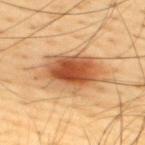Notes:
* biopsy status: imaged on a skin check; not biopsied
* subject: male, aged 43 to 47
* acquisition: ~15 mm tile from a whole-body skin photo
* automated lesion analysis: a lesion area of about 24 mm² and an outline eccentricity of about 0.8 (0 = round, 1 = elongated); border irregularity of about 3.5 on a 0–10 scale, a within-lesion color-variation index near 8.5/10, and peripheral color asymmetry of about 2; a nevus-likeness score of about 100/100 and a detector confidence of about 100 out of 100 that the crop contains a lesion
* body site: the upper back
* lesion size: ~7.5 mm (longest diameter)
* lighting: cross-polarized